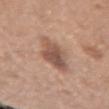biopsy status: imaged on a skin check; not biopsied.